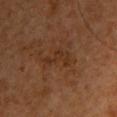Assessment:
Recorded during total-body skin imaging; not selected for excision or biopsy.
Clinical summary:
Measured at roughly 4 mm in maximum diameter. Located on the left upper arm. A 15 mm close-up tile from a total-body photography series done for melanoma screening. Captured under cross-polarized illumination. The subject is a male roughly 60 years of age.